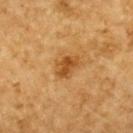Part of a total-body skin-imaging series; this lesion was reviewed on a skin check and was not flagged for biopsy. Located on the upper back. A male patient aged 83 or over. A close-up tile cropped from a whole-body skin photograph, about 15 mm across. The tile uses cross-polarized illumination.